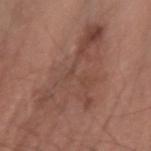Notes:
• workup — no biopsy performed (imaged during a skin exam)
• illumination — white-light
• lesion diameter — about 10 mm
• subject — male, aged 73 to 77
• acquisition — ~15 mm crop, total-body skin-cancer survey
• site — the arm